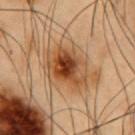A lesion tile, about 15 mm wide, cut from a 3D total-body photograph.
Captured under cross-polarized illumination.
About 5.5 mm across.
From the chest.
The total-body-photography lesion software estimated a shape eccentricity near 0.7 and a shape-asymmetry score of about 0.2 (0 = symmetric). And it measured a normalized lesion–skin contrast near 9.5.
A male subject aged 53–57.